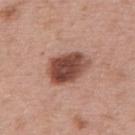Impression:
Recorded during total-body skin imaging; not selected for excision or biopsy.
Context:
The lesion's longest dimension is about 5 mm. Cropped from a whole-body photographic skin survey; the tile spans about 15 mm. The lesion-visualizer software estimated a lesion area of about 13 mm², a shape eccentricity near 0.7, and a shape-asymmetry score of about 0.15 (0 = symmetric). And it measured a lesion color around L≈46 a*≈23 b*≈27 in CIELAB, a lesion–skin lightness drop of about 17, and a normalized lesion–skin contrast near 12. It also reported a nevus-likeness score of about 85/100. This is a white-light tile. The lesion is on the upper back. A male subject in their mid-60s.